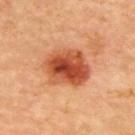A patient aged 63 to 67.
Imaged with cross-polarized lighting.
Longest diameter approximately 5.5 mm.
A region of skin cropped from a whole-body photographic capture, roughly 15 mm wide.
Automated tile analysis of the lesion measured an eccentricity of roughly 0.6 and two-axis asymmetry of about 0.15. And it measured about 16 CIELAB-L* units darker than the surrounding skin and a normalized lesion–skin contrast near 10.5. The analysis additionally found a border-irregularity index near 1.5/10 and internal color variation of about 9 on a 0–10 scale.
From the upper back.